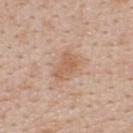Clinical impression: Part of a total-body skin-imaging series; this lesion was reviewed on a skin check and was not flagged for biopsy. Clinical summary: Captured under white-light illumination. A female patient, about 45 years old. On the upper back. This image is a 15 mm lesion crop taken from a total-body photograph. Measured at roughly 3.5 mm in maximum diameter.